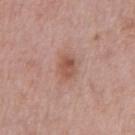{"patient": {"sex": "male", "age_approx": 70}, "site": "chest", "image": {"source": "total-body photography crop", "field_of_view_mm": 15}, "automated_metrics": {"cielab_L": 53, "cielab_a": 23, "cielab_b": 28, "nevus_likeness_0_100": 65}, "lighting": "white-light", "lesion_size": {"long_diameter_mm_approx": 2.5}}